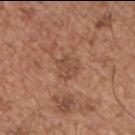Clinical impression: This lesion was catalogued during total-body skin photography and was not selected for biopsy. Background: A male subject, in their mid-50s. A 15 mm crop from a total-body photograph taken for skin-cancer surveillance. Measured at roughly 2.5 mm in maximum diameter. The lesion is located on the chest.